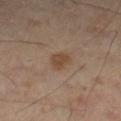{"biopsy_status": "not biopsied; imaged during a skin examination", "lesion_size": {"long_diameter_mm_approx": 3.0}, "site": "left leg", "patient": {"sex": "male", "age_approx": 50}, "automated_metrics": {"vs_skin_darker_L": 7.0, "vs_skin_contrast_norm": 7.0, "border_irregularity_0_10": 2.5, "color_variation_0_10": 2.5, "peripheral_color_asymmetry": 1.0}, "lighting": "cross-polarized", "image": {"source": "total-body photography crop", "field_of_view_mm": 15}}